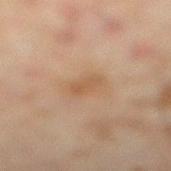Q: Was a biopsy performed?
A: imaged on a skin check; not biopsied
Q: Illumination type?
A: cross-polarized illumination
Q: What kind of image is this?
A: ~15 mm crop, total-body skin-cancer survey
Q: What is the anatomic site?
A: the leg
Q: Automated lesion metrics?
A: a mean CIELAB color near L≈47 a*≈17 b*≈30, roughly 6 lightness units darker than nearby skin, and a lesion-to-skin contrast of about 5.5 (normalized; higher = more distinct); border irregularity of about 3 on a 0–10 scale and internal color variation of about 1 on a 0–10 scale
Q: What are the patient's age and sex?
A: male, about 45 years old